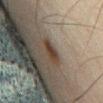notes = catalogued during a skin exam; not biopsied | lesion size = about 3 mm | anatomic site = the abdomen | patient = male, in their mid-40s | imaging modality = ~15 mm tile from a whole-body skin photo.